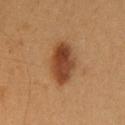Clinical impression:
The lesion was tiled from a total-body skin photograph and was not biopsied.
Acquisition and patient details:
Approximately 5.5 mm at its widest. On the upper back. The tile uses cross-polarized illumination. A region of skin cropped from a whole-body photographic capture, roughly 15 mm wide. A female subject, approximately 20 years of age.